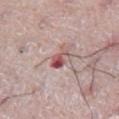follow-up: imaged on a skin check; not biopsied
body site: the abdomen
acquisition: 15 mm crop, total-body photography
diameter: ~3 mm (longest diameter)
patient: male, aged 73 to 77